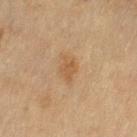Findings:
* biopsy status — imaged on a skin check; not biopsied